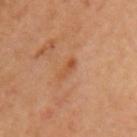workup=no biopsy performed (imaged during a skin exam)
TBP lesion metrics=a border-irregularity index near 4.5/10, a color-variation rating of about 0/10, and radial color variation of about 0
tile lighting=cross-polarized
location=the left upper arm
imaging modality=15 mm crop, total-body photography
subject=female, roughly 45 years of age
diameter=about 2.5 mm